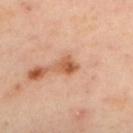A female patient, aged 38–42. A roughly 15 mm field-of-view crop from a total-body skin photograph. Located on the upper back. This is a cross-polarized tile. Approximately 2.5 mm at its widest.